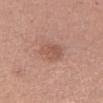Q: Was a biopsy performed?
A: no biopsy performed (imaged during a skin exam)
Q: What lighting was used for the tile?
A: white-light illumination
Q: Who is the patient?
A: female, in their 40s
Q: Lesion location?
A: the left forearm
Q: What did automated image analysis measure?
A: an average lesion color of about L≈54 a*≈22 b*≈27 (CIELAB) and a normalized lesion–skin contrast near 5.5
Q: What is the imaging modality?
A: ~15 mm crop, total-body skin-cancer survey
Q: Lesion size?
A: about 3 mm